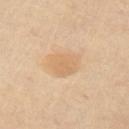biopsy status = catalogued during a skin exam; not biopsied.